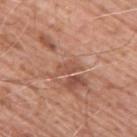| field | value |
|---|---|
| workup | imaged on a skin check; not biopsied |
| automated lesion analysis | a shape eccentricity near 0.9; a mean CIELAB color near L≈53 a*≈24 b*≈30 and about 7 CIELAB-L* units darker than the surrounding skin |
| patient | male, aged approximately 60 |
| anatomic site | the upper back |
| image | total-body-photography crop, ~15 mm field of view |
| illumination | white-light |